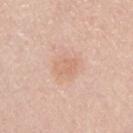Captured during whole-body skin photography for melanoma surveillance; the lesion was not biopsied. A male subject, aged approximately 40. A roughly 15 mm field-of-view crop from a total-body skin photograph. The lesion is located on the chest.